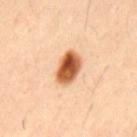Recorded during total-body skin imaging; not selected for excision or biopsy.
The tile uses cross-polarized illumination.
Cropped from a whole-body photographic skin survey; the tile spans about 15 mm.
The lesion's longest dimension is about 4 mm.
Located on the mid back.
A male subject aged approximately 40.